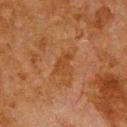{
  "biopsy_status": "not biopsied; imaged during a skin examination",
  "lighting": "cross-polarized",
  "automated_metrics": {
    "area_mm2_approx": 4.0,
    "eccentricity": 0.85,
    "shape_asymmetry": 0.35,
    "nevus_likeness_0_100": 0,
    "lesion_detection_confidence_0_100": 100
  },
  "site": "upper back",
  "lesion_size": {
    "long_diameter_mm_approx": 3.0
  },
  "image": {
    "source": "total-body photography crop",
    "field_of_view_mm": 15
  },
  "patient": {
    "sex": "male",
    "age_approx": 80
  }
}